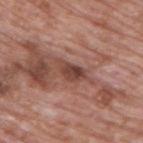Q: Was this lesion biopsied?
A: catalogued during a skin exam; not biopsied
Q: Lesion location?
A: the upper back
Q: Patient demographics?
A: male, aged around 70
Q: Automated lesion metrics?
A: a lesion area of about 5.5 mm², an outline eccentricity of about 0.9 (0 = round, 1 = elongated), and two-axis asymmetry of about 0.35; a classifier nevus-likeness of about 0/100
Q: How was this image acquired?
A: 15 mm crop, total-body photography